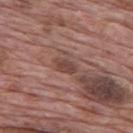This lesion was catalogued during total-body skin photography and was not selected for biopsy. The patient is a male about 70 years old. The lesion is on the mid back. Approximately 3 mm at its widest. This is a white-light tile. A region of skin cropped from a whole-body photographic capture, roughly 15 mm wide. The lesion-visualizer software estimated a mean CIELAB color near L≈44 a*≈21 b*≈23, roughly 9 lightness units darker than nearby skin, and a normalized border contrast of about 7. The analysis additionally found a color-variation rating of about 1.5/10 and peripheral color asymmetry of about 0.5.